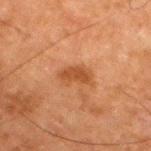notes: no biopsy performed (imaged during a skin exam)
automated lesion analysis: a lesion area of about 6 mm², a shape eccentricity near 0.85, and a shape-asymmetry score of about 0.25 (0 = symmetric); an average lesion color of about L≈40 a*≈22 b*≈33 (CIELAB), about 8 CIELAB-L* units darker than the surrounding skin, and a lesion-to-skin contrast of about 7 (normalized; higher = more distinct)
illumination: cross-polarized illumination
lesion size: ≈3.5 mm
image: ~15 mm crop, total-body skin-cancer survey
patient: male, aged 78 to 82
site: the right thigh